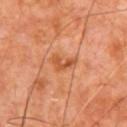Imaged during a routine full-body skin examination; the lesion was not biopsied and no histopathology is available.
From the chest.
A male subject, in their mid-60s.
A 15 mm crop from a total-body photograph taken for skin-cancer surveillance.
The total-body-photography lesion software estimated an area of roughly 4 mm², an outline eccentricity of about 0.85 (0 = round, 1 = elongated), and a symmetry-axis asymmetry near 0.6. And it measured an automated nevus-likeness rating near 0 out of 100 and a lesion-detection confidence of about 100/100.
Imaged with cross-polarized lighting.